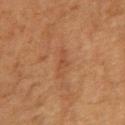Captured during whole-body skin photography for melanoma surveillance; the lesion was not biopsied. From the right upper arm. A 15 mm close-up tile from a total-body photography series done for melanoma screening. The lesion's longest dimension is about 4 mm. Captured under cross-polarized illumination. The patient is a female about 40 years old.